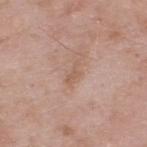Findings:
• follow-up: catalogued during a skin exam; not biopsied
• image: total-body-photography crop, ~15 mm field of view
• lighting: white-light
• patient: male, aged 53–57
• site: the upper back